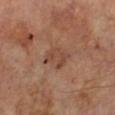Captured during whole-body skin photography for melanoma surveillance; the lesion was not biopsied. The lesion is on the left lower leg. This image is a 15 mm lesion crop taken from a total-body photograph. A male patient, roughly 70 years of age. About 4 mm across. The total-body-photography lesion software estimated an automated nevus-likeness rating near 0 out of 100 and lesion-presence confidence of about 100/100.